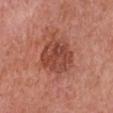Q: Is there a histopathology result?
A: no biopsy performed (imaged during a skin exam)
Q: What is the imaging modality?
A: ~15 mm crop, total-body skin-cancer survey
Q: What lighting was used for the tile?
A: white-light illumination
Q: What is the lesion's diameter?
A: ≈4.5 mm
Q: What are the patient's age and sex?
A: female, aged 58 to 62
Q: Where on the body is the lesion?
A: the front of the torso